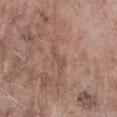notes: no biopsy performed (imaged during a skin exam)
acquisition: 15 mm crop, total-body photography
tile lighting: white-light illumination
location: the right forearm
patient: female, in their mid- to late 70s
diameter: ≈2.5 mm